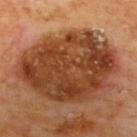Findings:
– follow-up · catalogued during a skin exam; not biopsied
– lesion diameter · about 11 mm
– automated metrics · a footprint of about 65 mm², an outline eccentricity of about 0.7 (0 = round, 1 = elongated), and a shape-asymmetry score of about 0.15 (0 = symmetric); about 14 CIELAB-L* units darker than the surrounding skin and a normalized lesion–skin contrast near 11; border irregularity of about 2 on a 0–10 scale, a within-lesion color-variation index near 6.5/10, and a peripheral color-asymmetry measure near 2.5; a classifier nevus-likeness of about 10/100 and a lesion-detection confidence of about 100/100
– patient · male, in their 60s
– image · ~15 mm tile from a whole-body skin photo
– tile lighting · cross-polarized
– location · the upper back